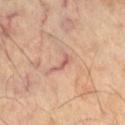biopsy status: no biopsy performed (imaged during a skin exam) | acquisition: 15 mm crop, total-body photography | lesion size: about 3 mm | body site: the right thigh | lighting: cross-polarized | patient: male, aged approximately 60.